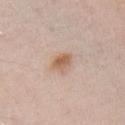This lesion was catalogued during total-body skin photography and was not selected for biopsy.
A male subject, about 70 years old.
This is a white-light tile.
The recorded lesion diameter is about 2.5 mm.
The lesion-visualizer software estimated a classifier nevus-likeness of about 85/100 and a detector confidence of about 100 out of 100 that the crop contains a lesion.
A close-up tile cropped from a whole-body skin photograph, about 15 mm across.
On the chest.